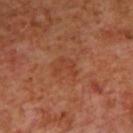Part of a total-body skin-imaging series; this lesion was reviewed on a skin check and was not flagged for biopsy. Measured at roughly 3 mm in maximum diameter. On the upper back. This is a cross-polarized tile. A 15 mm crop from a total-body photograph taken for skin-cancer surveillance. An algorithmic analysis of the crop reported an area of roughly 3.5 mm², an eccentricity of roughly 0.65, and two-axis asymmetry of about 0.65. A male subject approximately 70 years of age.